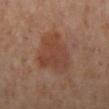| key | value |
|---|---|
| automated metrics | an area of roughly 18 mm² and a shape eccentricity near 0.65; an average lesion color of about L≈43 a*≈22 b*≈28 (CIELAB), roughly 8 lightness units darker than nearby skin, and a lesion-to-skin contrast of about 6.5 (normalized; higher = more distinct) |
| imaging modality | 15 mm crop, total-body photography |
| tile lighting | cross-polarized |
| subject | female, in their mid- to late 50s |
| anatomic site | the left lower leg |